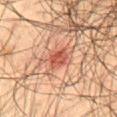No biopsy was performed on this lesion — it was imaged during a full skin examination and was not determined to be concerning.